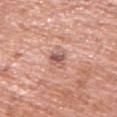workup — no biopsy performed (imaged during a skin exam); lighting — white-light; patient — male, aged 68–72; site — the right upper arm; image — 15 mm crop, total-body photography; lesion size — ≈3.5 mm.